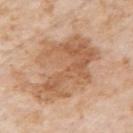Impression: This lesion was catalogued during total-body skin photography and was not selected for biopsy. Background: Approximately 9 mm at its widest. This image is a 15 mm lesion crop taken from a total-body photograph. Automated image analysis of the tile measured a footprint of about 34 mm², an outline eccentricity of about 0.75 (0 = round, 1 = elongated), and a symmetry-axis asymmetry near 0.4. The software also gave a lesion-detection confidence of about 100/100. The tile uses white-light illumination. The patient is a male approximately 75 years of age. The lesion is located on the right upper arm.